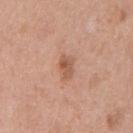Measured at roughly 3 mm in maximum diameter. A male subject aged 53–57. Imaged with white-light lighting. Cropped from a total-body skin-imaging series; the visible field is about 15 mm. The lesion is located on the abdomen.